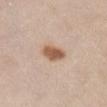Notes:
– location — the chest
– diameter — ~3.5 mm (longest diameter)
– imaging modality — 15 mm crop, total-body photography
– subject — female, roughly 50 years of age